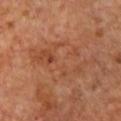Assessment:
Recorded during total-body skin imaging; not selected for excision or biopsy.